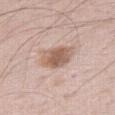subject: male, aged around 65
automated lesion analysis: a footprint of about 10 mm², an outline eccentricity of about 0.75 (0 = round, 1 = elongated), and two-axis asymmetry of about 0.25; a mean CIELAB color near L≈59 a*≈18 b*≈27, about 12 CIELAB-L* units darker than the surrounding skin, and a lesion-to-skin contrast of about 8 (normalized; higher = more distinct); a border-irregularity index near 2.5/10, a within-lesion color-variation index near 3.5/10, and a peripheral color-asymmetry measure near 1; lesion-presence confidence of about 100/100
acquisition: ~15 mm tile from a whole-body skin photo
location: the right thigh
tile lighting: white-light
diameter: ~4.5 mm (longest diameter)